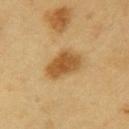| feature | finding |
|---|---|
| patient | male, aged approximately 60 |
| image source | ~15 mm tile from a whole-body skin photo |
| automated metrics | a mean CIELAB color near L≈54 a*≈19 b*≈42, about 12 CIELAB-L* units darker than the surrounding skin, and a lesion-to-skin contrast of about 9 (normalized; higher = more distinct); lesion-presence confidence of about 100/100 |
| illumination | cross-polarized |
| body site | the right upper arm |